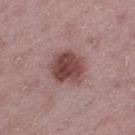<case>
<biopsy_status>not biopsied; imaged during a skin examination</biopsy_status>
<patient>
  <sex>female</sex>
  <age_approx>45</age_approx>
</patient>
<lighting>white-light</lighting>
<lesion_size>
  <long_diameter_mm_approx>4.5</long_diameter_mm_approx>
</lesion_size>
<site>left thigh</site>
<image>
  <source>total-body photography crop</source>
  <field_of_view_mm>15</field_of_view_mm>
</image>
</case>